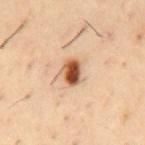Captured during whole-body skin photography for melanoma surveillance; the lesion was not biopsied. The tile uses cross-polarized illumination. Automated image analysis of the tile measured a footprint of about 5.5 mm², an outline eccentricity of about 0.65 (0 = round, 1 = elongated), and a symmetry-axis asymmetry near 0.2. The analysis additionally found an average lesion color of about L≈54 a*≈23 b*≈35 (CIELAB), a lesion–skin lightness drop of about 20, and a normalized border contrast of about 12.5. It also reported a within-lesion color-variation index near 9/10 and radial color variation of about 3. And it measured a nevus-likeness score of about 100/100. Cropped from a whole-body photographic skin survey; the tile spans about 15 mm. The recorded lesion diameter is about 3 mm. The lesion is on the mid back. A male patient aged approximately 55.